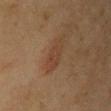workup — total-body-photography surveillance lesion; no biopsy | subject — female, about 55 years old | anatomic site — the right upper arm | image — 15 mm crop, total-body photography.